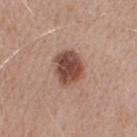Assessment: Part of a total-body skin-imaging series; this lesion was reviewed on a skin check and was not flagged for biopsy. Context: Cropped from a total-body skin-imaging series; the visible field is about 15 mm. The total-body-photography lesion software estimated a footprint of about 11 mm², an eccentricity of roughly 0.6, and a symmetry-axis asymmetry near 0.15. The software also gave a within-lesion color-variation index near 4.5/10 and radial color variation of about 1.5. It also reported an automated nevus-likeness rating near 80 out of 100 and lesion-presence confidence of about 100/100. Imaged with white-light lighting. Measured at roughly 4 mm in maximum diameter. The patient is a male about 50 years old. From the arm.